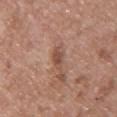Part of a total-body skin-imaging series; this lesion was reviewed on a skin check and was not flagged for biopsy. A male subject roughly 50 years of age. Located on the chest. A roughly 15 mm field-of-view crop from a total-body skin photograph.